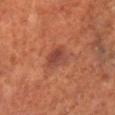Part of a total-body skin-imaging series; this lesion was reviewed on a skin check and was not flagged for biopsy. The subject is a male approximately 70 years of age. This image is a 15 mm lesion crop taken from a total-body photograph. The lesion is located on the right lower leg.